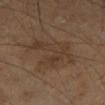{"site": "left thigh", "patient": {"sex": "male", "age_approx": 70}, "lesion_size": {"long_diameter_mm_approx": 7.0}, "image": {"source": "total-body photography crop", "field_of_view_mm": 15}, "lighting": "cross-polarized"}